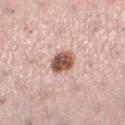Clinical impression: The lesion was photographed on a routine skin check and not biopsied; there is no pathology result.